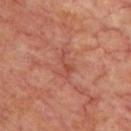The lesion is on the front of the torso.
A male subject approximately 65 years of age.
An algorithmic analysis of the crop reported a footprint of about 5 mm² and an outline eccentricity of about 0.9 (0 = round, 1 = elongated).
The tile uses cross-polarized illumination.
Cropped from a total-body skin-imaging series; the visible field is about 15 mm.